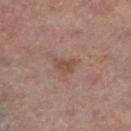Captured during whole-body skin photography for melanoma surveillance; the lesion was not biopsied. The lesion is on the left lower leg. A female patient roughly 65 years of age. The recorded lesion diameter is about 2.5 mm. A 15 mm close-up tile from a total-body photography series done for melanoma screening.